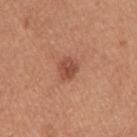The lesion was photographed on a routine skin check and not biopsied; there is no pathology result.
Automated tile analysis of the lesion measured roughly 10 lightness units darker than nearby skin and a normalized lesion–skin contrast near 7.5. The software also gave a nevus-likeness score of about 80/100 and a detector confidence of about 100 out of 100 that the crop contains a lesion.
About 2.5 mm across.
A close-up tile cropped from a whole-body skin photograph, about 15 mm across.
A female patient, aged 23 to 27.
Captured under white-light illumination.
From the right upper arm.